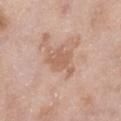biopsy status=no biopsy performed (imaged during a skin exam) | site=the right lower leg | subject=female, roughly 65 years of age | imaging modality=~15 mm crop, total-body skin-cancer survey.